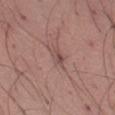Assessment: The lesion was photographed on a routine skin check and not biopsied; there is no pathology result. Clinical summary: Automated tile analysis of the lesion measured a nevus-likeness score of about 0/100 and a lesion-detection confidence of about 65/100. The lesion is on the left thigh. Captured under white-light illumination. The subject is a male roughly 20 years of age. A 15 mm crop from a total-body photograph taken for skin-cancer surveillance.